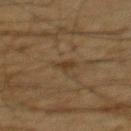Part of a total-body skin-imaging series; this lesion was reviewed on a skin check and was not flagged for biopsy.
The subject is a male about 60 years old.
The lesion is located on the mid back.
A region of skin cropped from a whole-body photographic capture, roughly 15 mm wide.